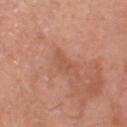{
  "biopsy_status": "not biopsied; imaged during a skin examination",
  "image": {
    "source": "total-body photography crop",
    "field_of_view_mm": 15
  },
  "site": "head or neck",
  "patient": {
    "sex": "male",
    "age_approx": 65
  },
  "lighting": "white-light"
}